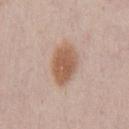follow-up: total-body-photography surveillance lesion; no biopsy | site: the chest | patient: male, aged around 35 | imaging modality: ~15 mm crop, total-body skin-cancer survey.